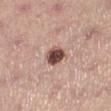{"biopsy_status": "not biopsied; imaged during a skin examination", "site": "leg", "image": {"source": "total-body photography crop", "field_of_view_mm": 15}, "patient": {"sex": "female", "age_approx": 65}, "automated_metrics": {"nevus_likeness_0_100": 95, "lesion_detection_confidence_0_100": 100}}